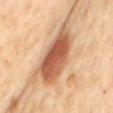Impression:
The lesion was photographed on a routine skin check and not biopsied; there is no pathology result.
Context:
Approximately 8 mm at its widest. A female patient in their mid-50s. A 15 mm close-up extracted from a 3D total-body photography capture. This is a cross-polarized tile. Located on the mid back. Automated image analysis of the tile measured a lesion–skin lightness drop of about 18 and a lesion-to-skin contrast of about 11 (normalized; higher = more distinct). The analysis additionally found a border-irregularity index near 3/10, a within-lesion color-variation index near 5.5/10, and peripheral color asymmetry of about 2. The analysis additionally found a detector confidence of about 100 out of 100 that the crop contains a lesion.